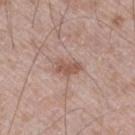notes: no biopsy performed (imaged during a skin exam)
illumination: white-light
patient: male, in their 70s
automated metrics: a mean CIELAB color near L≈55 a*≈20 b*≈26, a lesion–skin lightness drop of about 9, and a normalized border contrast of about 7; internal color variation of about 2.5 on a 0–10 scale and a peripheral color-asymmetry measure near 1; a nevus-likeness score of about 0/100
image source: total-body-photography crop, ~15 mm field of view
anatomic site: the right thigh